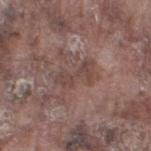{"biopsy_status": "not biopsied; imaged during a skin examination", "image": {"source": "total-body photography crop", "field_of_view_mm": 15}, "patient": {"sex": "male", "age_approx": 75}, "lesion_size": {"long_diameter_mm_approx": 4.5}, "lighting": "white-light", "site": "left thigh"}